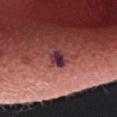Clinical impression: Imaged during a routine full-body skin examination; the lesion was not biopsied and no histopathology is available. Context: Longest diameter approximately 2.5 mm. The lesion is on the head or neck. A 15 mm close-up extracted from a 3D total-body photography capture. The patient is a male approximately 35 years of age. Imaged with white-light lighting.